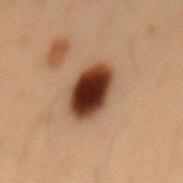Impression:
The lesion was tiled from a total-body skin photograph and was not biopsied.
Background:
A male patient approximately 55 years of age. A 15 mm close-up extracted from a 3D total-body photography capture. From the mid back.